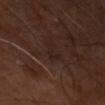Q: Was a biopsy performed?
A: no biopsy performed (imaged during a skin exam)
Q: What kind of image is this?
A: ~15 mm crop, total-body skin-cancer survey
Q: What did automated image analysis measure?
A: a footprint of about 4 mm², an eccentricity of roughly 0.65, and a shape-asymmetry score of about 0.6 (0 = symmetric); an average lesion color of about L≈21 a*≈16 b*≈18 (CIELAB), a lesion–skin lightness drop of about 4, and a lesion-to-skin contrast of about 5 (normalized; higher = more distinct); a border-irregularity index near 6/10, a within-lesion color-variation index near 2/10, and a peripheral color-asymmetry measure near 0.5; lesion-presence confidence of about 100/100
Q: Where on the body is the lesion?
A: the left upper arm
Q: What lighting was used for the tile?
A: cross-polarized
Q: What is the lesion's diameter?
A: ~2.5 mm (longest diameter)